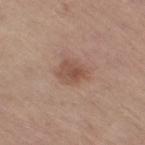– biopsy status · catalogued during a skin exam; not biopsied
– diameter · ≈3.5 mm
– body site · the right thigh
– image source · 15 mm crop, total-body photography
– subject · female, aged 63–67
– automated metrics · an average lesion color of about L≈51 a*≈20 b*≈27 (CIELAB), roughly 9 lightness units darker than nearby skin, and a normalized lesion–skin contrast near 7; a color-variation rating of about 3/10 and radial color variation of about 1; an automated nevus-likeness rating near 65 out of 100
– lighting · white-light